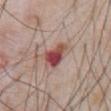| key | value |
|---|---|
| biopsy status | catalogued during a skin exam; not biopsied |
| lighting | white-light |
| lesion diameter | ~3.5 mm (longest diameter) |
| location | the abdomen |
| automated metrics | a mean CIELAB color near L≈48 a*≈27 b*≈23, a lesion–skin lightness drop of about 15, and a lesion-to-skin contrast of about 10.5 (normalized; higher = more distinct); a classifier nevus-likeness of about 0/100 and a detector confidence of about 100 out of 100 that the crop contains a lesion |
| acquisition | ~15 mm tile from a whole-body skin photo |
| patient | male, roughly 60 years of age |